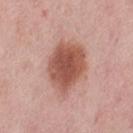notes — imaged on a skin check; not biopsied
size — about 6 mm
tile lighting — white-light illumination
automated lesion analysis — a footprint of about 22 mm², an eccentricity of roughly 0.65, and two-axis asymmetry of about 0.2; an automated nevus-likeness rating near 100 out of 100
body site — the left thigh
imaging modality — 15 mm crop, total-body photography
subject — female, about 50 years old